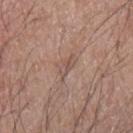biopsy status: total-body-photography surveillance lesion; no biopsy | patient: male, aged 63 to 67 | TBP lesion metrics: a lesion area of about 4.5 mm², an outline eccentricity of about 0.85 (0 = round, 1 = elongated), and two-axis asymmetry of about 0.45; a mean CIELAB color near L≈52 a*≈18 b*≈24, about 7 CIELAB-L* units darker than the surrounding skin, and a lesion-to-skin contrast of about 5 (normalized; higher = more distinct); a peripheral color-asymmetry measure near 1; an automated nevus-likeness rating near 0 out of 100 and a detector confidence of about 65 out of 100 that the crop contains a lesion | anatomic site: the left forearm | image: total-body-photography crop, ~15 mm field of view.